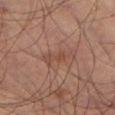Impression:
Imaged during a routine full-body skin examination; the lesion was not biopsied and no histopathology is available.
Image and clinical context:
A region of skin cropped from a whole-body photographic capture, roughly 15 mm wide. From the left thigh. Imaged with cross-polarized lighting. A male patient, aged 63–67. The lesion's longest dimension is about 4 mm.